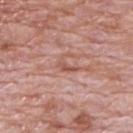notes: catalogued during a skin exam; not biopsied
lighting: white-light
image: ~15 mm tile from a whole-body skin photo
subject: male, roughly 70 years of age
anatomic site: the upper back
TBP lesion metrics: a lesion color around L≈55 a*≈24 b*≈27 in CIELAB and a normalized lesion–skin contrast near 6; a border-irregularity index near 6/10 and a color-variation rating of about 0.5/10